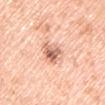Part of a total-body skin-imaging series; this lesion was reviewed on a skin check and was not flagged for biopsy. A lesion tile, about 15 mm wide, cut from a 3D total-body photograph. The lesion is on the left upper arm. The patient is a male in their 60s. The tile uses white-light illumination.